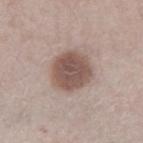Clinical impression: Part of a total-body skin-imaging series; this lesion was reviewed on a skin check and was not flagged for biopsy. Background: Imaged with white-light lighting. Measured at roughly 4.5 mm in maximum diameter. The subject is a male in their mid- to late 60s. A 15 mm crop from a total-body photograph taken for skin-cancer surveillance. Located on the right lower leg.